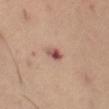The lesion was photographed on a routine skin check and not biopsied; there is no pathology result. A female subject, approximately 55 years of age. Imaged with cross-polarized lighting. On the abdomen. Longest diameter approximately 2.5 mm. A 15 mm close-up tile from a total-body photography series done for melanoma screening.